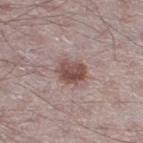The lesion is located on the right thigh.
A roughly 15 mm field-of-view crop from a total-body skin photograph.
Approximately 3.5 mm at its widest.
A male patient aged 48 to 52.
This is a white-light tile.
The total-body-photography lesion software estimated a border-irregularity rating of about 2/10 and a color-variation rating of about 5/10. It also reported an automated nevus-likeness rating near 70 out of 100 and lesion-presence confidence of about 100/100.